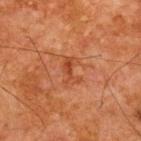workup: no biopsy performed (imaged during a skin exam) | TBP lesion metrics: an average lesion color of about L≈38 a*≈25 b*≈33 (CIELAB), a lesion–skin lightness drop of about 7, and a lesion-to-skin contrast of about 6 (normalized; higher = more distinct); border irregularity of about 6 on a 0–10 scale and internal color variation of about 1 on a 0–10 scale; an automated nevus-likeness rating near 0 out of 100 and a detector confidence of about 100 out of 100 that the crop contains a lesion | lesion size: about 3 mm | location: the upper back | tile lighting: cross-polarized | patient: male, aged around 65 | imaging modality: ~15 mm tile from a whole-body skin photo.